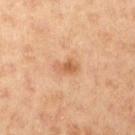Part of a total-body skin-imaging series; this lesion was reviewed on a skin check and was not flagged for biopsy. Located on the right upper arm. Captured under cross-polarized illumination. The total-body-photography lesion software estimated a lesion area of about 4 mm², a shape eccentricity near 0.8, and a symmetry-axis asymmetry near 0.35. It also reported an average lesion color of about L≈53 a*≈21 b*≈33 (CIELAB), about 9 CIELAB-L* units darker than the surrounding skin, and a normalized border contrast of about 6.5. And it measured an automated nevus-likeness rating near 35 out of 100. This image is a 15 mm lesion crop taken from a total-body photograph. A female subject approximately 20 years of age. About 3 mm across.